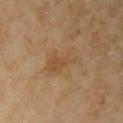The lesion was photographed on a routine skin check and not biopsied; there is no pathology result. The lesion is located on the right upper arm. A female patient roughly 60 years of age. A 15 mm crop from a total-body photograph taken for skin-cancer surveillance.